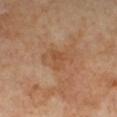Clinical impression:
This lesion was catalogued during total-body skin photography and was not selected for biopsy.
Image and clinical context:
Longest diameter approximately 2.5 mm. The tile uses cross-polarized illumination. The lesion-visualizer software estimated two-axis asymmetry of about 0.5. It also reported border irregularity of about 4.5 on a 0–10 scale and radial color variation of about 0.5. The software also gave an automated nevus-likeness rating near 0 out of 100 and a lesion-detection confidence of about 100/100. The subject is a female aged approximately 50. A 15 mm close-up extracted from a 3D total-body photography capture. On the left lower leg.